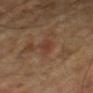biopsy_status: not biopsied; imaged during a skin examination